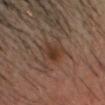No biopsy was performed on this lesion — it was imaged during a full skin examination and was not determined to be concerning.